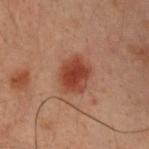notes=total-body-photography surveillance lesion; no biopsy | illumination=cross-polarized | body site=the back | image source=~15 mm tile from a whole-body skin photo | diameter=≈4.5 mm | subject=male, in their 30s | image-analysis metrics=a footprint of about 10 mm² and a symmetry-axis asymmetry near 0.2; a border-irregularity rating of about 2/10, a within-lesion color-variation index near 3/10, and a peripheral color-asymmetry measure near 1; an automated nevus-likeness rating near 100 out of 100 and lesion-presence confidence of about 100/100.